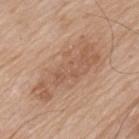subject: male, aged 78–82 | image: ~15 mm tile from a whole-body skin photo | illumination: white-light | size: about 9 mm | image-analysis metrics: an average lesion color of about L≈57 a*≈19 b*≈31 (CIELAB), a lesion–skin lightness drop of about 8, and a normalized border contrast of about 5.5; a classifier nevus-likeness of about 0/100 and lesion-presence confidence of about 100/100 | anatomic site: the chest.